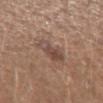Part of a total-body skin-imaging series; this lesion was reviewed on a skin check and was not flagged for biopsy.
A close-up tile cropped from a whole-body skin photograph, about 15 mm across.
An algorithmic analysis of the crop reported a lesion area of about 8 mm², a shape eccentricity near 0.65, and two-axis asymmetry of about 0.3. The analysis additionally found a mean CIELAB color near L≈47 a*≈17 b*≈24, about 8 CIELAB-L* units darker than the surrounding skin, and a normalized border contrast of about 6.5. And it measured a border-irregularity rating of about 3.5/10, internal color variation of about 5.5 on a 0–10 scale, and peripheral color asymmetry of about 2. The analysis additionally found a nevus-likeness score of about 15/100 and a detector confidence of about 100 out of 100 that the crop contains a lesion.
A female patient aged 38–42.
This is a white-light tile.
The lesion is on the left forearm.
The recorded lesion diameter is about 4 mm.